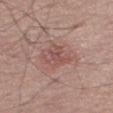Imaged during a routine full-body skin examination; the lesion was not biopsied and no histopathology is available. About 3.5 mm across. Cropped from a whole-body photographic skin survey; the tile spans about 15 mm. The tile uses white-light illumination. Automated image analysis of the tile measured a footprint of about 7.5 mm², an eccentricity of roughly 0.6, and two-axis asymmetry of about 0.45. And it measured a border-irregularity index near 6.5/10, a color-variation rating of about 3/10, and a peripheral color-asymmetry measure near 1. The software also gave lesion-presence confidence of about 100/100. The subject is a male aged around 70. From the right thigh.